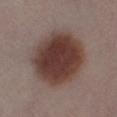illumination: cross-polarized illumination; size: ≈7 mm; site: the left lower leg; patient: male, approximately 40 years of age; image: ~15 mm crop, total-body skin-cancer survey.